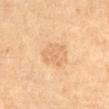Assessment:
Imaged during a routine full-body skin examination; the lesion was not biopsied and no histopathology is available.
Context:
An algorithmic analysis of the crop reported a mean CIELAB color near L≈68 a*≈19 b*≈39, roughly 7 lightness units darker than nearby skin, and a lesion-to-skin contrast of about 4.5 (normalized; higher = more distinct). The patient is a female aged 68–72. A 15 mm crop from a total-body photograph taken for skin-cancer surveillance. Imaged with cross-polarized lighting. Located on the right thigh. Longest diameter approximately 3.5 mm.